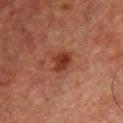Assessment: This lesion was catalogued during total-body skin photography and was not selected for biopsy. Context: This image is a 15 mm lesion crop taken from a total-body photograph. The subject is a male roughly 50 years of age. Imaged with cross-polarized lighting. An algorithmic analysis of the crop reported an eccentricity of roughly 0.6 and a symmetry-axis asymmetry near 0.35. The analysis additionally found a nevus-likeness score of about 75/100 and a detector confidence of about 100 out of 100 that the crop contains a lesion. The lesion is located on the chest. About 3 mm across.